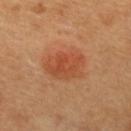{
  "biopsy_status": "not biopsied; imaged during a skin examination",
  "image": {
    "source": "total-body photography crop",
    "field_of_view_mm": 15
  },
  "patient": {
    "sex": "female",
    "age_approx": 60
  },
  "automated_metrics": {
    "area_mm2_approx": 13.0,
    "eccentricity": 0.75,
    "shape_asymmetry": 0.2,
    "cielab_L": 51,
    "cielab_a": 29,
    "cielab_b": 38,
    "vs_skin_darker_L": 9.0,
    "border_irregularity_0_10": 2.5,
    "color_variation_0_10": 4.0,
    "peripheral_color_asymmetry": 1.5,
    "nevus_likeness_0_100": 100,
    "lesion_detection_confidence_0_100": 100
  },
  "site": "back"
}